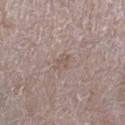Clinical impression: Recorded during total-body skin imaging; not selected for excision or biopsy. Context: The lesion's longest dimension is about 2.5 mm. A region of skin cropped from a whole-body photographic capture, roughly 15 mm wide. The lesion is on the right lower leg. The patient is a male in their 50s.